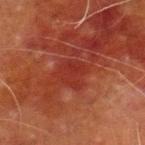workup — catalogued during a skin exam; not biopsied
imaging modality — total-body-photography crop, ~15 mm field of view
location — the left lower leg
automated lesion analysis — a lesion color around L≈29 a*≈30 b*≈28 in CIELAB and a lesion–skin lightness drop of about 5; a border-irregularity rating of about 3/10, a color-variation rating of about 2/10, and a peripheral color-asymmetry measure near 1; an automated nevus-likeness rating near 0 out of 100
subject — male, roughly 70 years of age